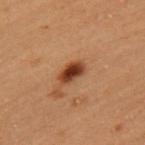{
  "biopsy_status": "not biopsied; imaged during a skin examination",
  "patient": {
    "sex": "female",
    "age_approx": 40
  },
  "lesion_size": {
    "long_diameter_mm_approx": 3.0
  },
  "image": {
    "source": "total-body photography crop",
    "field_of_view_mm": 15
  },
  "automated_metrics": {
    "area_mm2_approx": 5.5,
    "eccentricity": 0.75,
    "shape_asymmetry": 0.2,
    "color_variation_0_10": 5.0
  },
  "site": "left upper arm",
  "lighting": "cross-polarized"
}